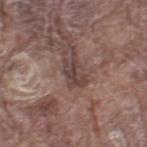A male patient, in their 80s. The lesion is located on the right thigh. Cropped from a whole-body photographic skin survey; the tile spans about 15 mm. The tile uses white-light illumination. Longest diameter approximately 3 mm.